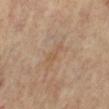workup = catalogued during a skin exam; not biopsied